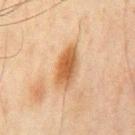Measured at roughly 5.5 mm in maximum diameter.
The lesion is located on the chest.
A male patient, roughly 45 years of age.
Imaged with cross-polarized lighting.
A roughly 15 mm field-of-view crop from a total-body skin photograph.